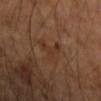Assessment:
Captured during whole-body skin photography for melanoma surveillance; the lesion was not biopsied.
Context:
Cropped from a total-body skin-imaging series; the visible field is about 15 mm. Measured at roughly 3.5 mm in maximum diameter. The subject is a female roughly 60 years of age. Captured under cross-polarized illumination. On the right forearm.